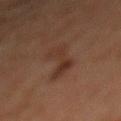Assessment:
Part of a total-body skin-imaging series; this lesion was reviewed on a skin check and was not flagged for biopsy.
Image and clinical context:
The tile uses cross-polarized illumination. The lesion is located on the front of the torso. The lesion-visualizer software estimated a lesion color around L≈30 a*≈17 b*≈24 in CIELAB and a lesion-to-skin contrast of about 6 (normalized; higher = more distinct). It also reported a nevus-likeness score of about 10/100. Cropped from a total-body skin-imaging series; the visible field is about 15 mm. The patient is a male aged 58 to 62. Measured at roughly 6 mm in maximum diameter.